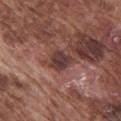| feature | finding |
|---|---|
| follow-up | total-body-photography surveillance lesion; no biopsy |
| subject | male, about 75 years old |
| lesion size | ≈2.5 mm |
| body site | the chest |
| tile lighting | white-light illumination |
| image source | 15 mm crop, total-body photography |